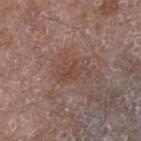Impression: This lesion was catalogued during total-body skin photography and was not selected for biopsy. Image and clinical context: A 15 mm close-up extracted from a 3D total-body photography capture. The lesion is on the right lower leg. This is a white-light tile. The total-body-photography lesion software estimated an area of roughly 5 mm² and a shape eccentricity near 0.7. And it measured a nevus-likeness score of about 0/100 and a lesion-detection confidence of about 100/100. A female patient, aged 73–77.